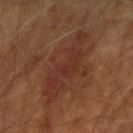| key | value |
|---|---|
| patient | male, roughly 70 years of age |
| TBP lesion metrics | about 5 CIELAB-L* units darker than the surrounding skin; a border-irregularity index near 6/10 and a color-variation rating of about 4.5/10 |
| site | the right forearm |
| imaging modality | total-body-photography crop, ~15 mm field of view |
| lighting | cross-polarized illumination |
| lesion size | ~8.5 mm (longest diameter) |